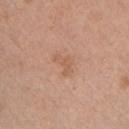{"biopsy_status": "not biopsied; imaged during a skin examination", "lesion_size": {"long_diameter_mm_approx": 3.0}, "lighting": "white-light", "patient": {"sex": "male", "age_approx": 30}, "site": "front of the torso", "image": {"source": "total-body photography crop", "field_of_view_mm": 15}}